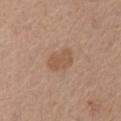| feature | finding |
|---|---|
| automated lesion analysis | an average lesion color of about L≈54 a*≈18 b*≈30 (CIELAB) and roughly 8 lightness units darker than nearby skin; a border-irregularity rating of about 2.5/10, a color-variation rating of about 2.5/10, and peripheral color asymmetry of about 1 |
| acquisition | ~15 mm tile from a whole-body skin photo |
| body site | the abdomen |
| size | ~3.5 mm (longest diameter) |
| patient | male, in their mid-70s |